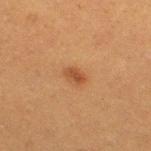Captured during whole-body skin photography for melanoma surveillance; the lesion was not biopsied. A female subject approximately 40 years of age. On the right thigh. A close-up tile cropped from a whole-body skin photograph, about 15 mm across.